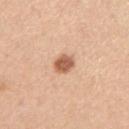No biopsy was performed on this lesion — it was imaged during a full skin examination and was not determined to be concerning. The recorded lesion diameter is about 2.5 mm. This image is a 15 mm lesion crop taken from a total-body photograph. From the right upper arm. A female patient, aged 38 to 42.